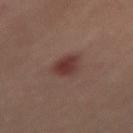Imaged during a routine full-body skin examination; the lesion was not biopsied and no histopathology is available.
A close-up tile cropped from a whole-body skin photograph, about 15 mm across.
Imaged with cross-polarized lighting.
The lesion is on the left thigh.
The recorded lesion diameter is about 3.5 mm.
A female patient, approximately 45 years of age.